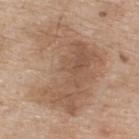This lesion was catalogued during total-body skin photography and was not selected for biopsy.
From the upper back.
A male subject aged approximately 75.
Cropped from a whole-body photographic skin survey; the tile spans about 15 mm.
The tile uses white-light illumination.
The recorded lesion diameter is about 10.5 mm.
Automated tile analysis of the lesion measured an outline eccentricity of about 0.7 (0 = round, 1 = elongated) and a symmetry-axis asymmetry near 0.45. The software also gave a lesion color around L≈56 a*≈17 b*≈30 in CIELAB, roughly 9 lightness units darker than nearby skin, and a normalized border contrast of about 6.5.